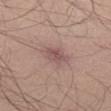notes: imaged on a skin check; not biopsied
patient: male, aged 63–67
location: the left thigh
image source: ~15 mm tile from a whole-body skin photo